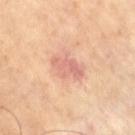<tbp_lesion>
<biopsy_status>not biopsied; imaged during a skin examination</biopsy_status>
<lighting>cross-polarized</lighting>
<patient>
  <sex>male</sex>
  <age_approx>65</age_approx>
</patient>
<image>
  <source>total-body photography crop</source>
  <field_of_view_mm>15</field_of_view_mm>
</image>
<lesion_size>
  <long_diameter_mm_approx>4.0</long_diameter_mm_approx>
</lesion_size>
<automated_metrics>
  <eccentricity>0.8</eccentricity>
  <lesion_detection_confidence_0_100>100</lesion_detection_confidence_0_100>
</automated_metrics>
</tbp_lesion>